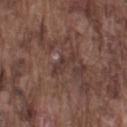Impression: Imaged during a routine full-body skin examination; the lesion was not biopsied and no histopathology is available. Context: The lesion's longest dimension is about 3.5 mm. The lesion is located on the left upper arm. Automated tile analysis of the lesion measured border irregularity of about 6.5 on a 0–10 scale and a peripheral color-asymmetry measure near 0. And it measured a nevus-likeness score of about 0/100 and a detector confidence of about 55 out of 100 that the crop contains a lesion. Imaged with white-light lighting. A region of skin cropped from a whole-body photographic capture, roughly 15 mm wide. A male subject aged approximately 75.